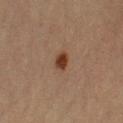biopsy status = total-body-photography surveillance lesion; no biopsy
image source = 15 mm crop, total-body photography
patient = female, in their mid-60s
lesion size = ≈2.5 mm
site = the left upper arm
illumination = cross-polarized illumination